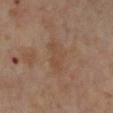<tbp_lesion>
<biopsy_status>not biopsied; imaged during a skin examination</biopsy_status>
<patient>
  <sex>female</sex>
  <age_approx>55</age_approx>
</patient>
<site>left lower leg</site>
<lesion_size>
  <long_diameter_mm_approx>4.5</long_diameter_mm_approx>
</lesion_size>
<image>
  <source>total-body photography crop</source>
  <field_of_view_mm>15</field_of_view_mm>
</image>
</tbp_lesion>